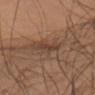The lesion was photographed on a routine skin check and not biopsied; there is no pathology result. On the left arm. A male subject, aged around 50. A 15 mm close-up tile from a total-body photography series done for melanoma screening. Imaged with cross-polarized lighting.